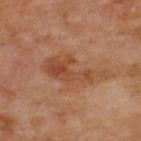<case>
  <biopsy_status>not biopsied; imaged during a skin examination</biopsy_status>
  <automated_metrics>
    <cielab_L>46</cielab_L>
    <cielab_a>22</cielab_a>
    <cielab_b>33</cielab_b>
    <vs_skin_contrast_norm>6.5</vs_skin_contrast_norm>
    <border_irregularity_0_10>7.0</border_irregularity_0_10>
    <color_variation_0_10>4.5</color_variation_0_10>
    <peripheral_color_asymmetry>1.5</peripheral_color_asymmetry>
  </automated_metrics>
  <patient>
    <sex>male</sex>
    <age_approx>70</age_approx>
  </patient>
  <image>
    <source>total-body photography crop</source>
    <field_of_view_mm>15</field_of_view_mm>
  </image>
  <lighting>cross-polarized</lighting>
  <site>upper back</site>
</case>